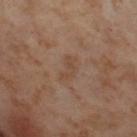| field | value |
|---|---|
| follow-up | total-body-photography surveillance lesion; no biopsy |
| imaging modality | total-body-photography crop, ~15 mm field of view |
| image-analysis metrics | a lesion area of about 4 mm², an eccentricity of roughly 0.85, and a shape-asymmetry score of about 0.4 (0 = symmetric); border irregularity of about 4.5 on a 0–10 scale and internal color variation of about 0.5 on a 0–10 scale; a classifier nevus-likeness of about 0/100 and a detector confidence of about 100 out of 100 that the crop contains a lesion |
| lighting | cross-polarized illumination |
| anatomic site | the left thigh |
| lesion diameter | ≈3 mm |
| patient | female, aged 53 to 57 |